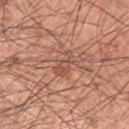A male subject aged 58–62. An algorithmic analysis of the crop reported a mean CIELAB color near L≈52 a*≈24 b*≈30, a lesion–skin lightness drop of about 8, and a lesion-to-skin contrast of about 5.5 (normalized; higher = more distinct). And it measured a within-lesion color-variation index near 2/10 and radial color variation of about 0.5. And it measured a nevus-likeness score of about 0/100 and lesion-presence confidence of about 90/100. A 15 mm close-up extracted from a 3D total-body photography capture. Approximately 3.5 mm at its widest. Captured under white-light illumination. From the left upper arm.